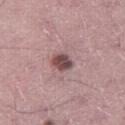notes: imaged on a skin check; not biopsied | acquisition: ~15 mm tile from a whole-body skin photo | patient: male, aged 43 to 47 | body site: the right thigh.